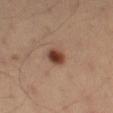A region of skin cropped from a whole-body photographic capture, roughly 15 mm wide.
The tile uses cross-polarized illumination.
The lesion is on the leg.
The total-body-photography lesion software estimated a mean CIELAB color near L≈41 a*≈22 b*≈29, roughly 15 lightness units darker than nearby skin, and a lesion-to-skin contrast of about 12 (normalized; higher = more distinct). And it measured a border-irregularity rating of about 2/10, a within-lesion color-variation index near 4/10, and a peripheral color-asymmetry measure near 1. And it measured a nevus-likeness score of about 100/100 and a detector confidence of about 100 out of 100 that the crop contains a lesion.
A male subject about 40 years old.
Measured at roughly 2.5 mm in maximum diameter.